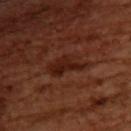A region of skin cropped from a whole-body photographic capture, roughly 15 mm wide. This is a cross-polarized tile. Measured at roughly 5 mm in maximum diameter. The lesion is on the upper back. A female patient about 55 years old.